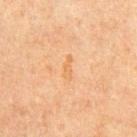{
  "biopsy_status": "not biopsied; imaged during a skin examination",
  "image": {
    "source": "total-body photography crop",
    "field_of_view_mm": 15
  },
  "patient": {
    "sex": "male",
    "age_approx": 65
  },
  "site": "chest"
}